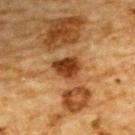Captured during whole-body skin photography for melanoma surveillance; the lesion was not biopsied.
Approximately 3.5 mm at its widest.
The tile uses cross-polarized illumination.
Cropped from a total-body skin-imaging series; the visible field is about 15 mm.
The total-body-photography lesion software estimated a lesion area of about 7.5 mm² and an outline eccentricity of about 0.6 (0 = round, 1 = elongated). The software also gave a lesion color around L≈36 a*≈22 b*≈35 in CIELAB, roughly 14 lightness units darker than nearby skin, and a lesion-to-skin contrast of about 11 (normalized; higher = more distinct). The software also gave a color-variation rating of about 4/10 and a peripheral color-asymmetry measure near 1.5.
From the upper back.
A male subject about 85 years old or older.